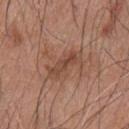The lesion was photographed on a routine skin check and not biopsied; there is no pathology result. The lesion is on the upper back. A male patient, in their mid- to late 40s. A region of skin cropped from a whole-body photographic capture, roughly 15 mm wide. Imaged with white-light lighting.